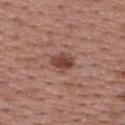<lesion>
  <biopsy_status>not biopsied; imaged during a skin examination</biopsy_status>
  <site>upper back</site>
  <lighting>white-light</lighting>
  <patient>
    <sex>female</sex>
    <age_approx>50</age_approx>
  </patient>
  <automated_metrics>
    <area_mm2_approx>5.0</area_mm2_approx>
    <eccentricity>0.7</eccentricity>
    <shape_asymmetry>0.15</shape_asymmetry>
    <nevus_likeness_0_100>90</nevus_likeness_0_100>
    <lesion_detection_confidence_0_100>100</lesion_detection_confidence_0_100>
  </automated_metrics>
  <image>
    <source>total-body photography crop</source>
    <field_of_view_mm>15</field_of_view_mm>
  </image>
</lesion>